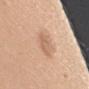This lesion was catalogued during total-body skin photography and was not selected for biopsy. A female patient in their mid-40s. Cropped from a total-body skin-imaging series; the visible field is about 15 mm. This is a white-light tile. The lesion is on the right upper arm.